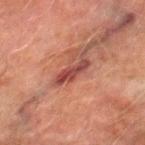* location · the right thigh
* tile lighting · cross-polarized illumination
* patient · male, aged 73 to 77
* acquisition · total-body-photography crop, ~15 mm field of view
* image-analysis metrics · border irregularity of about 3.5 on a 0–10 scale, a color-variation rating of about 2.5/10, and a peripheral color-asymmetry measure near 0.5; a lesion-detection confidence of about 85/100
* lesion diameter · ~4 mm (longest diameter)